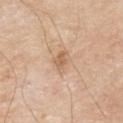Clinical impression:
No biopsy was performed on this lesion — it was imaged during a full skin examination and was not determined to be concerning.
Background:
Automated image analysis of the tile measured a border-irregularity index near 3/10 and a peripheral color-asymmetry measure near 0.5. Cropped from a total-body skin-imaging series; the visible field is about 15 mm. The lesion is on the right upper arm. About 2.5 mm across. A male subject, aged 78–82.